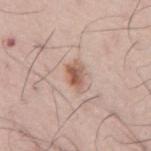Recorded during total-body skin imaging; not selected for excision or biopsy. The tile uses white-light illumination. The lesion's longest dimension is about 3.5 mm. Located on the mid back. A male patient, in their mid-70s. A 15 mm close-up tile from a total-body photography series done for melanoma screening.